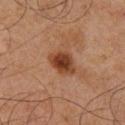  biopsy_status: not biopsied; imaged during a skin examination
  lesion_size:
    long_diameter_mm_approx: 3.5
  patient:
    sex: male
    age_approx: 65
  automated_metrics:
    area_mm2_approx: 7.0
    eccentricity: 0.7
    shape_asymmetry: 0.25
    border_irregularity_0_10: 2.0
    color_variation_0_10: 3.5
    peripheral_color_asymmetry: 1.0
    nevus_likeness_0_100: 95
    lesion_detection_confidence_0_100: 100
  site: right lower leg
  lighting: cross-polarized
  image:
    source: total-body photography crop
    field_of_view_mm: 15